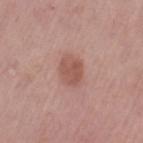Imaged during a routine full-body skin examination; the lesion was not biopsied and no histopathology is available.
A 15 mm crop from a total-body photograph taken for skin-cancer surveillance.
Automated tile analysis of the lesion measured a lesion area of about 7.5 mm², an outline eccentricity of about 0.65 (0 = round, 1 = elongated), and a shape-asymmetry score of about 0.2 (0 = symmetric). The analysis additionally found a lesion color around L≈54 a*≈23 b*≈26 in CIELAB, a lesion–skin lightness drop of about 9, and a lesion-to-skin contrast of about 6.5 (normalized; higher = more distinct). The analysis additionally found a color-variation rating of about 2.5/10. It also reported an automated nevus-likeness rating near 65 out of 100 and a detector confidence of about 100 out of 100 that the crop contains a lesion.
A female patient about 65 years old.
The lesion is located on the left thigh.
This is a white-light tile.